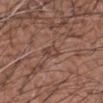No biopsy was performed on this lesion — it was imaged during a full skin examination and was not determined to be concerning.
The tile uses white-light illumination.
Located on the left forearm.
A close-up tile cropped from a whole-body skin photograph, about 15 mm across.
Approximately 2.5 mm at its widest.
Automated image analysis of the tile measured a lesion color around L≈43 a*≈19 b*≈25 in CIELAB, about 7 CIELAB-L* units darker than the surrounding skin, and a lesion-to-skin contrast of about 6 (normalized; higher = more distinct). And it measured a within-lesion color-variation index near 3.5/10 and a peripheral color-asymmetry measure near 1.5. And it measured a classifier nevus-likeness of about 0/100.
A male patient roughly 60 years of age.